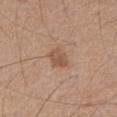| feature | finding |
|---|---|
| biopsy status | total-body-photography surveillance lesion; no biopsy |
| tile lighting | white-light |
| anatomic site | the front of the torso |
| patient | male, in their mid-70s |
| imaging modality | ~15 mm crop, total-body skin-cancer survey |
| diameter | about 2.5 mm |